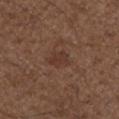Clinical impression: Imaged during a routine full-body skin examination; the lesion was not biopsied and no histopathology is available. Image and clinical context: Automated tile analysis of the lesion measured a mean CIELAB color near L≈35 a*≈19 b*≈24. It also reported internal color variation of about 1 on a 0–10 scale and radial color variation of about 0.5. The analysis additionally found an automated nevus-likeness rating near 15 out of 100 and a detector confidence of about 100 out of 100 that the crop contains a lesion. Measured at roughly 2.5 mm in maximum diameter. From the right upper arm. The patient is a male roughly 50 years of age. Imaged with white-light lighting. Cropped from a total-body skin-imaging series; the visible field is about 15 mm.